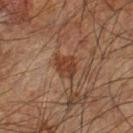{"biopsy_status": "not biopsied; imaged during a skin examination", "image": {"source": "total-body photography crop", "field_of_view_mm": 15}, "site": "arm", "patient": {"sex": "male", "age_approx": 65}}